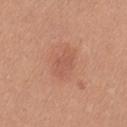The lesion was photographed on a routine skin check and not biopsied; there is no pathology result. The tile uses white-light illumination. A female patient approximately 35 years of age. Automated tile analysis of the lesion measured a symmetry-axis asymmetry near 0.15. And it measured a mean CIELAB color near L≈56 a*≈25 b*≈31 and a lesion–skin lightness drop of about 7. It also reported a border-irregularity rating of about 2.5/10 and internal color variation of about 1.5 on a 0–10 scale. It also reported a nevus-likeness score of about 20/100 and a detector confidence of about 100 out of 100 that the crop contains a lesion. Approximately 4 mm at its widest. From the left thigh. A roughly 15 mm field-of-view crop from a total-body skin photograph.